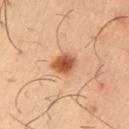Q: How was this image acquired?
A: ~15 mm tile from a whole-body skin photo
Q: Automated lesion metrics?
A: a footprint of about 6 mm², a shape eccentricity near 0.55, and a shape-asymmetry score of about 0.2 (0 = symmetric); about 16 CIELAB-L* units darker than the surrounding skin and a lesion-to-skin contrast of about 10.5 (normalized; higher = more distinct); a border-irregularity index near 2/10, a within-lesion color-variation index near 6/10, and radial color variation of about 2; an automated nevus-likeness rating near 100 out of 100 and a detector confidence of about 100 out of 100 that the crop contains a lesion
Q: What is the anatomic site?
A: the right upper arm
Q: What lighting was used for the tile?
A: cross-polarized illumination
Q: Patient demographics?
A: male, in their 40s